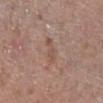The lesion was tiled from a total-body skin photograph and was not biopsied.
A female subject, aged around 65.
Approximately 3.5 mm at its widest.
A 15 mm close-up extracted from a 3D total-body photography capture.
From the right lower leg.
Captured under white-light illumination.